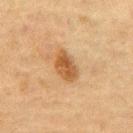workup = catalogued during a skin exam; not biopsied
subject = male, aged approximately 75
diameter = ~4 mm (longest diameter)
tile lighting = cross-polarized
site = the front of the torso
image source = total-body-photography crop, ~15 mm field of view